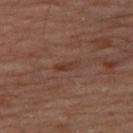follow-up = imaged on a skin check; not biopsied | acquisition = ~15 mm crop, total-body skin-cancer survey | tile lighting = cross-polarized | site = the right thigh | patient = male, aged around 70 | image-analysis metrics = a lesion area of about 2.5 mm², a shape eccentricity near 0.9, and two-axis asymmetry of about 0.25; border irregularity of about 2.5 on a 0–10 scale, a color-variation rating of about 0/10, and peripheral color asymmetry of about 0.